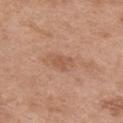Case summary:
• follow-up · catalogued during a skin exam; not biopsied
• image-analysis metrics · a lesion color around L≈55 a*≈22 b*≈31 in CIELAB, roughly 7 lightness units darker than nearby skin, and a lesion-to-skin contrast of about 5.5 (normalized; higher = more distinct); a border-irregularity index near 4/10, a color-variation rating of about 0.5/10, and peripheral color asymmetry of about 0; an automated nevus-likeness rating near 0 out of 100 and a detector confidence of about 100 out of 100 that the crop contains a lesion
• subject · female, about 65 years old
• imaging modality · 15 mm crop, total-body photography
• tile lighting · white-light
• anatomic site · the chest